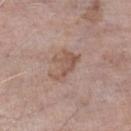Case summary:
• biopsy status: imaged on a skin check; not biopsied
• site: the leg
• patient: male, about 75 years old
• tile lighting: white-light
• image: ~15 mm tile from a whole-body skin photo
• TBP lesion metrics: an eccentricity of roughly 0.8; a lesion color around L≈54 a*≈18 b*≈26 in CIELAB and a lesion-to-skin contrast of about 6.5 (normalized; higher = more distinct); border irregularity of about 7.5 on a 0–10 scale and internal color variation of about 2.5 on a 0–10 scale; a detector confidence of about 100 out of 100 that the crop contains a lesion